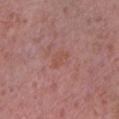Clinical impression:
Recorded during total-body skin imaging; not selected for excision or biopsy.
Context:
This image is a 15 mm lesion crop taken from a total-body photograph. A female subject, aged around 40. Captured under white-light illumination. Approximately 2.5 mm at its widest. Located on the right forearm.